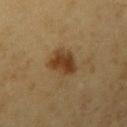follow-up: catalogued during a skin exam; not biopsied
image: 15 mm crop, total-body photography
site: the arm
lesion diameter: about 4 mm
TBP lesion metrics: a lesion area of about 8 mm², an outline eccentricity of about 0.75 (0 = round, 1 = elongated), and a symmetry-axis asymmetry near 0.2; a border-irregularity index near 2.5/10, a color-variation rating of about 3.5/10, and a peripheral color-asymmetry measure near 1
illumination: cross-polarized
patient: male, aged 58 to 62